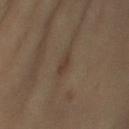Recorded during total-body skin imaging; not selected for excision or biopsy.